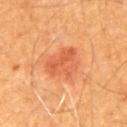Imaged during a routine full-body skin examination; the lesion was not biopsied and no histopathology is available.
On the chest.
A male patient aged 58–62.
The recorded lesion diameter is about 4.5 mm.
A region of skin cropped from a whole-body photographic capture, roughly 15 mm wide.